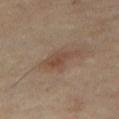The lesion was photographed on a routine skin check and not biopsied; there is no pathology result.
Approximately 4.5 mm at its widest.
This is a cross-polarized tile.
A female patient, in their 50s.
A close-up tile cropped from a whole-body skin photograph, about 15 mm across.
The lesion is located on the leg.
The lesion-visualizer software estimated an area of roughly 6.5 mm², a shape eccentricity near 0.9, and a symmetry-axis asymmetry near 0.35. It also reported a lesion-detection confidence of about 100/100.